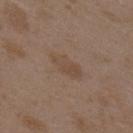This lesion was catalogued during total-body skin photography and was not selected for biopsy.
A 15 mm close-up tile from a total-body photography series done for melanoma screening.
On the back.
A female subject in their mid-30s.
Automated tile analysis of the lesion measured an area of roughly 6.5 mm² and a shape-asymmetry score of about 0.2 (0 = symmetric). And it measured an average lesion color of about L≈46 a*≈14 b*≈26 (CIELAB), about 6 CIELAB-L* units darker than the surrounding skin, and a normalized border contrast of about 5.
The tile uses white-light illumination.
Longest diameter approximately 4 mm.